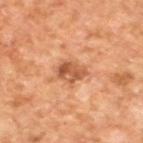Notes:
* follow-up · imaged on a skin check; not biopsied
* body site · the left lower leg
* size · ~3.5 mm (longest diameter)
* subject · male, aged 58–62
* image · 15 mm crop, total-body photography